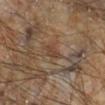Findings:
- follow-up — imaged on a skin check; not biopsied
- location — the right lower leg
- patient — male, aged around 60
- automated metrics — a lesion color around L≈36 a*≈16 b*≈25 in CIELAB, roughly 6 lightness units darker than nearby skin, and a normalized lesion–skin contrast near 6; a classifier nevus-likeness of about 0/100 and lesion-presence confidence of about 90/100
- lesion size — ~2 mm (longest diameter)
- imaging modality — total-body-photography crop, ~15 mm field of view